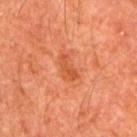The lesion was tiled from a total-body skin photograph and was not biopsied.
Approximately 3 mm at its widest.
This is a cross-polarized tile.
A male patient, in their mid-60s.
From the right upper arm.
This image is a 15 mm lesion crop taken from a total-body photograph.